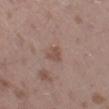Recorded during total-body skin imaging; not selected for excision or biopsy. On the left thigh. This is a white-light tile. A male patient, aged approximately 45. A close-up tile cropped from a whole-body skin photograph, about 15 mm across. The recorded lesion diameter is about 2.5 mm.